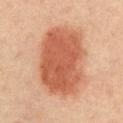Q: Is there a histopathology result?
A: catalogued during a skin exam; not biopsied
Q: How was this image acquired?
A: ~15 mm tile from a whole-body skin photo
Q: Patient demographics?
A: male, approximately 70 years of age
Q: What is the anatomic site?
A: the abdomen